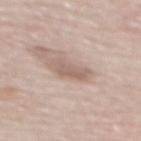{"biopsy_status": "not biopsied; imaged during a skin examination", "lesion_size": {"long_diameter_mm_approx": 3.5}, "lighting": "white-light", "patient": {"sex": "female", "age_approx": 65}, "image": {"source": "total-body photography crop", "field_of_view_mm": 15}, "site": "upper back"}